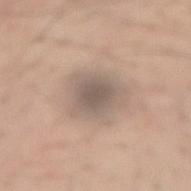<lesion>
<biopsy_status>not biopsied; imaged during a skin examination</biopsy_status>
<patient>
  <sex>male</sex>
  <age_approx>55</age_approx>
</patient>
<image>
  <source>total-body photography crop</source>
  <field_of_view_mm>15</field_of_view_mm>
</image>
<site>right forearm</site>
<lighting>white-light</lighting>
<lesion_size>
  <long_diameter_mm_approx>4.5</long_diameter_mm_approx>
</lesion_size>
</lesion>